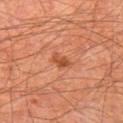Q: What did automated image analysis measure?
A: an area of roughly 2.5 mm², an outline eccentricity of about 0.8 (0 = round, 1 = elongated), and two-axis asymmetry of about 0.25; border irregularity of about 3 on a 0–10 scale, a color-variation rating of about 0.5/10, and a peripheral color-asymmetry measure near 0
Q: What is the lesion's diameter?
A: about 2.5 mm
Q: Who is the patient?
A: male, aged 63–67
Q: How was this image acquired?
A: 15 mm crop, total-body photography
Q: Where on the body is the lesion?
A: the left thigh